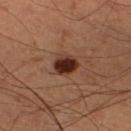Part of a total-body skin-imaging series; this lesion was reviewed on a skin check and was not flagged for biopsy.
A 15 mm close-up extracted from a 3D total-body photography capture.
Longest diameter approximately 3 mm.
A male subject roughly 60 years of age.
Automated image analysis of the tile measured about 16 CIELAB-L* units darker than the surrounding skin. And it measured a classifier nevus-likeness of about 95/100 and a lesion-detection confidence of about 100/100.
From the leg.
The tile uses cross-polarized illumination.